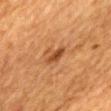Part of a total-body skin-imaging series; this lesion was reviewed on a skin check and was not flagged for biopsy. The lesion-visualizer software estimated an outline eccentricity of about 0.85 (0 = round, 1 = elongated) and a shape-asymmetry score of about 0.4 (0 = symmetric). It also reported an average lesion color of about L≈40 a*≈22 b*≈34 (CIELAB) and a normalized lesion–skin contrast near 8. The software also gave a nevus-likeness score of about 55/100 and lesion-presence confidence of about 100/100. A 15 mm close-up extracted from a 3D total-body photography capture. Located on the mid back. Measured at roughly 3 mm in maximum diameter. A female subject, approximately 55 years of age.